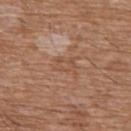Imaged during a routine full-body skin examination; the lesion was not biopsied and no histopathology is available. This is a white-light tile. The lesion's longest dimension is about 3 mm. A roughly 15 mm field-of-view crop from a total-body skin photograph. The subject is a male aged 58 to 62. From the chest.